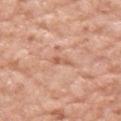{"image": {"source": "total-body photography crop", "field_of_view_mm": 15}, "automated_metrics": {"area_mm2_approx": 2.0, "eccentricity": 0.95, "cielab_L": 60, "cielab_a": 24, "cielab_b": 33, "vs_skin_darker_L": 9.0, "vs_skin_contrast_norm": 6.0, "border_irregularity_0_10": 4.0, "color_variation_0_10": 0.0, "peripheral_color_asymmetry": 0.0, "nevus_likeness_0_100": 0, "lesion_detection_confidence_0_100": 80}, "site": "right upper arm", "lesion_size": {"long_diameter_mm_approx": 2.5}, "patient": {"sex": "female", "age_approx": 75}, "lighting": "white-light"}